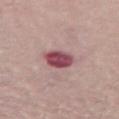Recorded during total-body skin imaging; not selected for excision or biopsy. On the right thigh. A close-up tile cropped from a whole-body skin photograph, about 15 mm across. A female patient about 70 years old. Longest diameter approximately 3.5 mm. Imaged with white-light lighting.